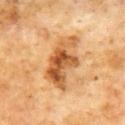The lesion was photographed on a routine skin check and not biopsied; there is no pathology result. A 15 mm crop from a total-body photograph taken for skin-cancer surveillance. Located on the chest. The subject is a male roughly 60 years of age.